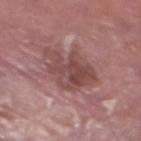This lesion was catalogued during total-body skin photography and was not selected for biopsy. A 15 mm crop from a total-body photograph taken for skin-cancer surveillance. Longest diameter approximately 5.5 mm. A male subject, in their 40s. Located on the left lower leg.